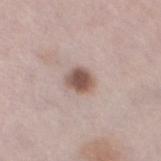biopsy status = total-body-photography surveillance lesion; no biopsy
subject = male, roughly 65 years of age
imaging modality = total-body-photography crop, ~15 mm field of view
site = the left thigh
lesion diameter = about 3 mm
lighting = white-light